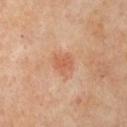Case summary:
- biopsy status — imaged on a skin check; not biopsied
- location — the front of the torso
- imaging modality — 15 mm crop, total-body photography
- subject — female, aged approximately 65